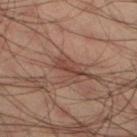Part of a total-body skin-imaging series; this lesion was reviewed on a skin check and was not flagged for biopsy. A lesion tile, about 15 mm wide, cut from a 3D total-body photograph. The lesion is on the left lower leg. The subject is a male aged 33 to 37. The tile uses cross-polarized illumination. The total-body-photography lesion software estimated a footprint of about 3 mm², an eccentricity of roughly 0.95, and a shape-asymmetry score of about 0.45 (0 = symmetric). The software also gave a lesion color around L≈37 a*≈20 b*≈23 in CIELAB and a normalized border contrast of about 7.5. The software also gave border irregularity of about 5.5 on a 0–10 scale, internal color variation of about 0.5 on a 0–10 scale, and a peripheral color-asymmetry measure near 0. And it measured an automated nevus-likeness rating near 5 out of 100 and a detector confidence of about 50 out of 100 that the crop contains a lesion. Approximately 3.5 mm at its widest.